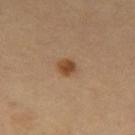Impression: No biopsy was performed on this lesion — it was imaged during a full skin examination and was not determined to be concerning.